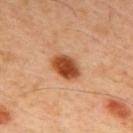<case>
  <biopsy_status>not biopsied; imaged during a skin examination</biopsy_status>
  <lighting>cross-polarized</lighting>
  <automated_metrics>
    <eccentricity>0.7</eccentricity>
    <shape_asymmetry>0.15</shape_asymmetry>
  </automated_metrics>
  <lesion_size>
    <long_diameter_mm_approx>4.0</long_diameter_mm_approx>
  </lesion_size>
  <patient>
    <sex>male</sex>
    <age_approx>45</age_approx>
  </patient>
  <site>upper back</site>
  <image>
    <source>total-body photography crop</source>
    <field_of_view_mm>15</field_of_view_mm>
  </image>
</case>